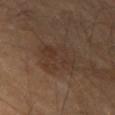No biopsy was performed on this lesion — it was imaged during a full skin examination and was not determined to be concerning.
The lesion's longest dimension is about 4 mm.
Imaged with cross-polarized lighting.
From the left forearm.
This image is a 15 mm lesion crop taken from a total-body photograph.
Automated image analysis of the tile measured a lesion area of about 12 mm², an outline eccentricity of about 0.45 (0 = round, 1 = elongated), and a shape-asymmetry score of about 0.25 (0 = symmetric). The software also gave a mean CIELAB color near L≈32 a*≈15 b*≈23, about 5 CIELAB-L* units darker than the surrounding skin, and a normalized border contrast of about 5. The analysis additionally found a nevus-likeness score of about 5/100 and lesion-presence confidence of about 100/100.
A male patient aged 68 to 72.